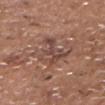workup = imaged on a skin check; not biopsied | size = about 5 mm | image-analysis metrics = border irregularity of about 6.5 on a 0–10 scale and radial color variation of about 2 | patient = male, aged around 70 | image = total-body-photography crop, ~15 mm field of view | body site = the chest.